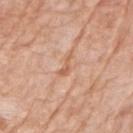notes = imaged on a skin check; not biopsied
body site = the left upper arm
image source = ~15 mm tile from a whole-body skin photo
subject = female, aged 73–77